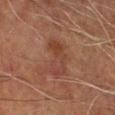notes — total-body-photography surveillance lesion; no biopsy | patient — male, aged approximately 70 | body site — the left lower leg | diameter — ≈4.5 mm | image — total-body-photography crop, ~15 mm field of view.